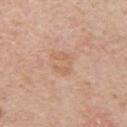Imaged during a routine full-body skin examination; the lesion was not biopsied and no histopathology is available. An algorithmic analysis of the crop reported a footprint of about 5 mm², a shape eccentricity near 0.75, and two-axis asymmetry of about 0.45. It also reported roughly 6 lightness units darker than nearby skin. Captured under white-light illumination. A male patient, in their mid-60s. The lesion is located on the left upper arm. A region of skin cropped from a whole-body photographic capture, roughly 15 mm wide. About 3 mm across.